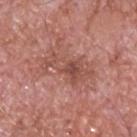follow-up = catalogued during a skin exam; not biopsied
patient = male, roughly 60 years of age
image source = total-body-photography crop, ~15 mm field of view
location = the upper back